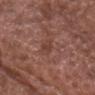Case summary:
* notes · imaged on a skin check; not biopsied
* lesion diameter · ≈3 mm
* subject · male, roughly 75 years of age
* image · 15 mm crop, total-body photography
* illumination · white-light illumination
* site · the chest